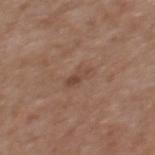workup — total-body-photography surveillance lesion; no biopsy
patient — male, aged 63–67
automated metrics — a lesion color around L≈45 a*≈19 b*≈27 in CIELAB and a normalized lesion–skin contrast near 5.5; border irregularity of about 3 on a 0–10 scale, a color-variation rating of about 3/10, and radial color variation of about 1; a nevus-likeness score of about 0/100 and lesion-presence confidence of about 100/100
tile lighting — white-light
acquisition — 15 mm crop, total-body photography
location — the mid back